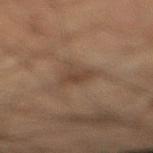Recorded during total-body skin imaging; not selected for excision or biopsy. A close-up tile cropped from a whole-body skin photograph, about 15 mm across. The subject is a male aged approximately 50. An algorithmic analysis of the crop reported a lesion area of about 4.5 mm² and a shape-asymmetry score of about 0.3 (0 = symmetric). The software also gave a mean CIELAB color near L≈31 a*≈12 b*≈21. From the right lower leg. The tile uses cross-polarized illumination. Approximately 3 mm at its widest.